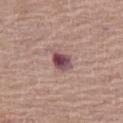Assessment:
Captured during whole-body skin photography for melanoma surveillance; the lesion was not biopsied.
Acquisition and patient details:
A 15 mm crop from a total-body photograph taken for skin-cancer surveillance. Located on the leg. The subject is a female roughly 65 years of age.